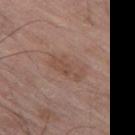biopsy status: imaged on a skin check; not biopsied
anatomic site: the left thigh
illumination: white-light
patient: male, aged approximately 80
lesion size: about 4.5 mm
image-analysis metrics: a within-lesion color-variation index near 3/10 and radial color variation of about 1; a classifier nevus-likeness of about 0/100
imaging modality: total-body-photography crop, ~15 mm field of view